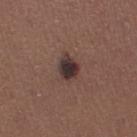Approximately 3 mm at its widest. The subject is a female aged 43 to 47. The lesion-visualizer software estimated a mean CIELAB color near L≈32 a*≈15 b*≈16 and a normalized lesion–skin contrast near 13. The analysis additionally found an automated nevus-likeness rating near 25 out of 100 and a detector confidence of about 100 out of 100 that the crop contains a lesion. The lesion is located on the right thigh. A close-up tile cropped from a whole-body skin photograph, about 15 mm across. Imaged with white-light lighting.